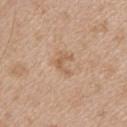| key | value |
|---|---|
| biopsy status | total-body-photography surveillance lesion; no biopsy |
| illumination | white-light |
| site | the upper back |
| imaging modality | ~15 mm tile from a whole-body skin photo |
| patient | female, roughly 45 years of age |
| automated lesion analysis | a footprint of about 4.5 mm² and a shape eccentricity near 0.65; a mean CIELAB color near L≈60 a*≈18 b*≈33 and a lesion-to-skin contrast of about 5.5 (normalized; higher = more distinct); border irregularity of about 7 on a 0–10 scale, a color-variation rating of about 0.5/10, and peripheral color asymmetry of about 0; a classifier nevus-likeness of about 0/100 and a detector confidence of about 100 out of 100 that the crop contains a lesion |